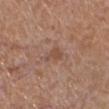The lesion was photographed on a routine skin check and not biopsied; there is no pathology result. A 15 mm close-up tile from a total-body photography series done for melanoma screening. Imaged with white-light lighting. A female patient, in their mid-50s. Located on the right lower leg.